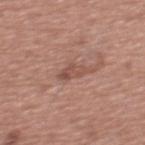notes = total-body-photography surveillance lesion; no biopsy | lighting = white-light | subject = male, approximately 45 years of age | acquisition = ~15 mm tile from a whole-body skin photo | lesion size = ≈3 mm | TBP lesion metrics = a mean CIELAB color near L≈50 a*≈22 b*≈26, about 8 CIELAB-L* units darker than the surrounding skin, and a normalized border contrast of about 6; a border-irregularity index near 3.5/10, a within-lesion color-variation index near 3.5/10, and radial color variation of about 1.5; a classifier nevus-likeness of about 0/100 and lesion-presence confidence of about 100/100 | site = the chest.